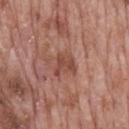Case summary:
- follow-up: imaged on a skin check; not biopsied
- image source: ~15 mm tile from a whole-body skin photo
- illumination: white-light
- subject: male, about 70 years old
- location: the mid back